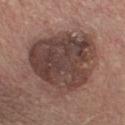Findings:
- notes — total-body-photography surveillance lesion; no biopsy
- acquisition — ~15 mm crop, total-body skin-cancer survey
- tile lighting — white-light illumination
- subject — male, roughly 60 years of age
- anatomic site — the front of the torso
- lesion size — ~8.5 mm (longest diameter)
- automated lesion analysis — an average lesion color of about L≈41 a*≈17 b*≈21 (CIELAB) and a lesion-to-skin contrast of about 9.5 (normalized; higher = more distinct); a border-irregularity rating of about 2.5/10, internal color variation of about 6.5 on a 0–10 scale, and a peripheral color-asymmetry measure near 2